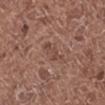- workup · no biopsy performed (imaged during a skin exam)
- patient · female, aged approximately 50
- lesion diameter · ≈3.5 mm
- body site · the right lower leg
- acquisition · total-body-photography crop, ~15 mm field of view